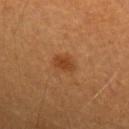Captured under cross-polarized illumination. A female patient approximately 50 years of age. A lesion tile, about 15 mm wide, cut from a 3D total-body photograph. The lesion's longest dimension is about 2.5 mm. The lesion is on the left forearm.